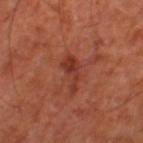Q: Is there a histopathology result?
A: imaged on a skin check; not biopsied
Q: Lesion location?
A: the left thigh
Q: Automated lesion metrics?
A: a lesion color around L≈36 a*≈30 b*≈31 in CIELAB and a lesion-to-skin contrast of about 7 (normalized; higher = more distinct); a within-lesion color-variation index near 2/10
Q: What lighting was used for the tile?
A: cross-polarized illumination
Q: How large is the lesion?
A: ≈4.5 mm
Q: What kind of image is this?
A: total-body-photography crop, ~15 mm field of view
Q: Who is the patient?
A: about 65 years old